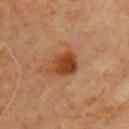{
  "site": "right upper arm",
  "lighting": "cross-polarized",
  "image": {
    "source": "total-body photography crop",
    "field_of_view_mm": 15
  },
  "automated_metrics": {
    "area_mm2_approx": 7.5,
    "eccentricity": 0.6,
    "shape_asymmetry": 0.3,
    "border_irregularity_0_10": 3.0,
    "color_variation_0_10": 3.5,
    "peripheral_color_asymmetry": 1.5
  },
  "patient": {
    "sex": "male",
    "age_approx": 70
  }
}